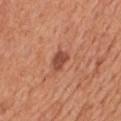* illumination · white-light
* patient · male, aged approximately 65
* anatomic site · the front of the torso
* diameter · ~2.5 mm (longest diameter)
* image · total-body-photography crop, ~15 mm field of view